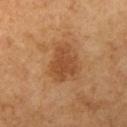Notes:
* follow-up: catalogued during a skin exam; not biopsied
* automated metrics: a lesion area of about 11 mm², an outline eccentricity of about 0.65 (0 = round, 1 = elongated), and two-axis asymmetry of about 0.3; a lesion color around L≈41 a*≈20 b*≈33 in CIELAB, about 8 CIELAB-L* units darker than the surrounding skin, and a lesion-to-skin contrast of about 7 (normalized; higher = more distinct); a nevus-likeness score of about 15/100 and lesion-presence confidence of about 100/100
* site: the left upper arm
* size: about 4.5 mm
* tile lighting: cross-polarized illumination
* acquisition: 15 mm crop, total-body photography
* patient: female, aged 58–62